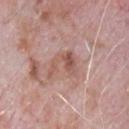The lesion was photographed on a routine skin check and not biopsied; there is no pathology result. A male subject, approximately 65 years of age. Measured at roughly 4.5 mm in maximum diameter. From the chest. The total-body-photography lesion software estimated a shape eccentricity near 0.8. The software also gave a lesion color around L≈54 a*≈21 b*≈25 in CIELAB and a lesion–skin lightness drop of about 9. The software also gave a border-irregularity rating of about 8.5/10, a within-lesion color-variation index near 5/10, and a peripheral color-asymmetry measure near 2. And it measured a nevus-likeness score of about 0/100 and a detector confidence of about 95 out of 100 that the crop contains a lesion. A 15 mm close-up extracted from a 3D total-body photography capture.